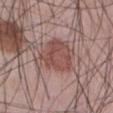Clinical impression:
Part of a total-body skin-imaging series; this lesion was reviewed on a skin check and was not flagged for biopsy.
Background:
About 5 mm across. A male subject aged 43 to 47. On the abdomen. This image is a 15 mm lesion crop taken from a total-body photograph.